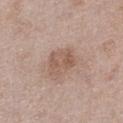Q: Was this lesion biopsied?
A: total-body-photography surveillance lesion; no biopsy